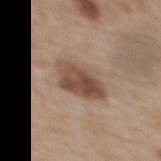{"image": {"source": "total-body photography crop", "field_of_view_mm": 15}, "site": "upper back", "automated_metrics": {"vs_skin_contrast_norm": 9.0, "border_irregularity_0_10": 2.5, "color_variation_0_10": 6.0, "peripheral_color_asymmetry": 2.5, "nevus_likeness_0_100": 80, "lesion_detection_confidence_0_100": 100}, "lighting": "white-light", "patient": {"sex": "female", "age_approx": 65}, "lesion_size": {"long_diameter_mm_approx": 5.5}}